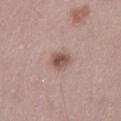A 15 mm crop from a total-body photograph taken for skin-cancer surveillance.
A female patient, in their 30s.
Located on the left thigh.